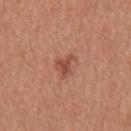Clinical summary: This is a white-light tile. A male patient aged approximately 65. Cropped from a total-body skin-imaging series; the visible field is about 15 mm. About 2.5 mm across. From the mid back.